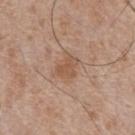Captured during whole-body skin photography for melanoma surveillance; the lesion was not biopsied. The lesion is located on the chest. Approximately 3 mm at its widest. Cropped from a total-body skin-imaging series; the visible field is about 15 mm. Captured under white-light illumination. A male patient approximately 65 years of age.